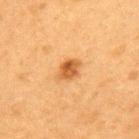Q: Where on the body is the lesion?
A: the upper back
Q: Who is the patient?
A: female, aged 38 to 42
Q: How was this image acquired?
A: ~15 mm tile from a whole-body skin photo